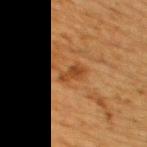Imaged during a routine full-body skin examination; the lesion was not biopsied and no histopathology is available. Automated image analysis of the tile measured a footprint of about 3.5 mm², a shape eccentricity near 0.9, and two-axis asymmetry of about 0.35. It also reported a lesion color around L≈37 a*≈22 b*≈35 in CIELAB, roughly 8 lightness units darker than nearby skin, and a normalized border contrast of about 7. It also reported border irregularity of about 4 on a 0–10 scale and a color-variation rating of about 1/10. It also reported a classifier nevus-likeness of about 10/100. Cropped from a whole-body photographic skin survey; the tile spans about 15 mm. Measured at roughly 3 mm in maximum diameter. From the upper back. The patient is a male aged 58–62. The tile uses cross-polarized illumination.